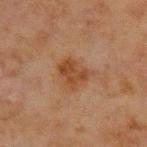The lesion was tiled from a total-body skin photograph and was not biopsied. The tile uses cross-polarized illumination. Located on the chest. A region of skin cropped from a whole-body photographic capture, roughly 15 mm wide. The lesion-visualizer software estimated a lesion color around L≈36 a*≈19 b*≈30 in CIELAB, about 7 CIELAB-L* units darker than the surrounding skin, and a normalized lesion–skin contrast near 8. The recorded lesion diameter is about 3.5 mm. A male patient, in their mid-60s.